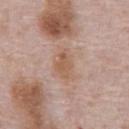automated metrics — a lesion area of about 5.5 mm² and a shape-asymmetry score of about 0.25 (0 = symmetric); a border-irregularity index near 3/10, a color-variation rating of about 2/10, and peripheral color asymmetry of about 0.5
imaging modality — ~15 mm tile from a whole-body skin photo
patient — male, about 70 years old
anatomic site — the chest
tile lighting — white-light